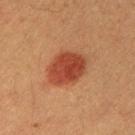An algorithmic analysis of the crop reported a border-irregularity index near 1.5/10, internal color variation of about 3.5 on a 0–10 scale, and peripheral color asymmetry of about 1. It also reported an automated nevus-likeness rating near 100 out of 100 and lesion-presence confidence of about 100/100. A region of skin cropped from a whole-body photographic capture, roughly 15 mm wide. The subject is a male aged approximately 40. This is a cross-polarized tile. On the right upper arm.